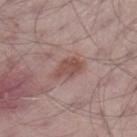Q: Is there a histopathology result?
A: catalogued during a skin exam; not biopsied
Q: Lesion size?
A: ≈4 mm
Q: Patient demographics?
A: male, aged around 65
Q: What is the anatomic site?
A: the left thigh
Q: Automated lesion metrics?
A: a lesion area of about 7.5 mm² and a symmetry-axis asymmetry near 0.25; an average lesion color of about L≈50 a*≈20 b*≈22 (CIELAB) and roughly 9 lightness units darker than nearby skin; a border-irregularity rating of about 2.5/10, a within-lesion color-variation index near 2.5/10, and peripheral color asymmetry of about 1; an automated nevus-likeness rating near 65 out of 100 and lesion-presence confidence of about 100/100
Q: How was the tile lit?
A: white-light
Q: What kind of image is this?
A: 15 mm crop, total-body photography